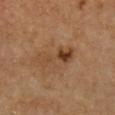patient: female, in their 50s | acquisition: ~15 mm crop, total-body skin-cancer survey | size: about 5.5 mm | body site: the chest | TBP lesion metrics: an outline eccentricity of about 0.95 (0 = round, 1 = elongated) and two-axis asymmetry of about 0.35; an average lesion color of about L≈42 a*≈20 b*≈32 (CIELAB), a lesion–skin lightness drop of about 8, and a normalized border contrast of about 7; a border-irregularity rating of about 6/10 and internal color variation of about 6.5 on a 0–10 scale | lighting: cross-polarized.